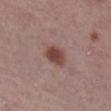Q: Was a biopsy performed?
A: no biopsy performed (imaged during a skin exam)
Q: How was the tile lit?
A: white-light illumination
Q: What did automated image analysis measure?
A: a lesion area of about 6 mm² and an outline eccentricity of about 0.65 (0 = round, 1 = elongated); a within-lesion color-variation index near 3/10 and a peripheral color-asymmetry measure near 1
Q: What is the lesion's diameter?
A: ≈3 mm
Q: What are the patient's age and sex?
A: female, about 65 years old
Q: How was this image acquired?
A: total-body-photography crop, ~15 mm field of view
Q: Where on the body is the lesion?
A: the leg